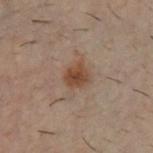Impression: Captured during whole-body skin photography for melanoma surveillance; the lesion was not biopsied. Clinical summary: Automated image analysis of the tile measured a lesion area of about 5 mm², an eccentricity of roughly 0.55, and a symmetry-axis asymmetry near 0.25. The software also gave a lesion color around L≈35 a*≈15 b*≈24 in CIELAB, a lesion–skin lightness drop of about 8, and a normalized border contrast of about 8.5. It also reported a classifier nevus-likeness of about 90/100 and lesion-presence confidence of about 100/100. The tile uses cross-polarized illumination. Approximately 2.5 mm at its widest. A 15 mm close-up tile from a total-body photography series done for melanoma screening. The lesion is located on the chest. The subject is a male in their 30s.